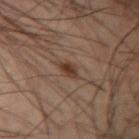{"biopsy_status": "not biopsied; imaged during a skin examination", "patient": {"sex": "male", "age_approx": 45}, "site": "left forearm", "automated_metrics": {"cielab_L": 35, "cielab_a": 16, "cielab_b": 25, "vs_skin_darker_L": 8.0, "vs_skin_contrast_norm": 8.0, "color_variation_0_10": 3.0, "peripheral_color_asymmetry": 1.0}, "lesion_size": {"long_diameter_mm_approx": 3.0}, "image": {"source": "total-body photography crop", "field_of_view_mm": 15}, "lighting": "cross-polarized"}